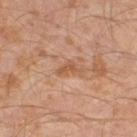This lesion was catalogued during total-body skin photography and was not selected for biopsy.
The lesion is on the left leg.
The total-body-photography lesion software estimated a footprint of about 3 mm², an eccentricity of roughly 0.85, and a shape-asymmetry score of about 0.6 (0 = symmetric). The software also gave a lesion color around L≈53 a*≈22 b*≈33 in CIELAB and roughly 8 lightness units darker than nearby skin. It also reported a lesion-detection confidence of about 100/100.
A male subject, aged approximately 30.
A close-up tile cropped from a whole-body skin photograph, about 15 mm across.